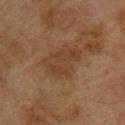Captured during whole-body skin photography for melanoma surveillance; the lesion was not biopsied. The patient is a male in their mid- to late 70s. The lesion-visualizer software estimated an area of roughly 11 mm², an eccentricity of roughly 0.8, and a shape-asymmetry score of about 0.3 (0 = symmetric). The software also gave a border-irregularity rating of about 4/10 and a color-variation rating of about 2.5/10. The software also gave a classifier nevus-likeness of about 0/100 and a detector confidence of about 100 out of 100 that the crop contains a lesion. From the upper back. A 15 mm close-up tile from a total-body photography series done for melanoma screening. Measured at roughly 5 mm in maximum diameter.